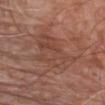Imaged during a routine full-body skin examination; the lesion was not biopsied and no histopathology is available. From the chest. A male subject, aged 63–67. An algorithmic analysis of the crop reported a lesion color around L≈45 a*≈22 b*≈27 in CIELAB, roughly 6 lightness units darker than nearby skin, and a lesion-to-skin contrast of about 5 (normalized; higher = more distinct). It also reported a classifier nevus-likeness of about 0/100. Cropped from a total-body skin-imaging series; the visible field is about 15 mm.